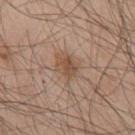This lesion was catalogued during total-body skin photography and was not selected for biopsy. Captured under white-light illumination. Automated image analysis of the tile measured a footprint of about 5 mm², a shape eccentricity near 0.4, and a symmetry-axis asymmetry near 0.2. The software also gave a mean CIELAB color near L≈50 a*≈17 b*≈29 and a lesion-to-skin contrast of about 7 (normalized; higher = more distinct). And it measured lesion-presence confidence of about 100/100. A roughly 15 mm field-of-view crop from a total-body skin photograph. A male subject about 45 years old. The lesion's longest dimension is about 2.5 mm. From the upper back.